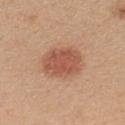Recorded during total-body skin imaging; not selected for excision or biopsy.
The lesion is on the upper back.
Cropped from a total-body skin-imaging series; the visible field is about 15 mm.
A female subject aged 23 to 27.